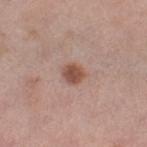<tbp_lesion>
  <biopsy_status>not biopsied; imaged during a skin examination</biopsy_status>
  <site>right thigh</site>
  <automated_metrics>
    <cielab_L>51</cielab_L>
    <cielab_a>21</cielab_a>
    <cielab_b>27</cielab_b>
    <vs_skin_darker_L>11.0</vs_skin_darker_L>
    <vs_skin_contrast_norm>8.5</vs_skin_contrast_norm>
  </automated_metrics>
  <patient>
    <sex>female</sex>
    <age_approx>50</age_approx>
  </patient>
  <image>
    <source>total-body photography crop</source>
    <field_of_view_mm>15</field_of_view_mm>
  </image>
  <lesion_size>
    <long_diameter_mm_approx>2.5</long_diameter_mm_approx>
  </lesion_size>
</tbp_lesion>